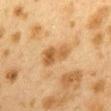Impression: The lesion was tiled from a total-body skin photograph and was not biopsied. Image and clinical context: The lesion's longest dimension is about 4.5 mm. The lesion-visualizer software estimated a lesion area of about 8 mm², a shape eccentricity near 0.85, and two-axis asymmetry of about 0.3. And it measured a mean CIELAB color near L≈49 a*≈17 b*≈36, roughly 9 lightness units darker than nearby skin, and a normalized border contrast of about 7.5. The analysis additionally found border irregularity of about 3.5 on a 0–10 scale, internal color variation of about 5 on a 0–10 scale, and peripheral color asymmetry of about 1.5. The analysis additionally found a nevus-likeness score of about 0/100 and a lesion-detection confidence of about 100/100. A female subject, aged approximately 40. A roughly 15 mm field-of-view crop from a total-body skin photograph. Located on the mid back.